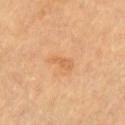Findings:
* biopsy status: imaged on a skin check; not biopsied
* lesion diameter: about 3 mm
* lighting: cross-polarized
* acquisition: ~15 mm tile from a whole-body skin photo
* subject: male, in their mid- to late 80s or older
* anatomic site: the chest